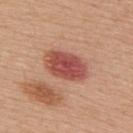This is a white-light tile. A close-up tile cropped from a whole-body skin photograph, about 15 mm across. Measured at roughly 5.5 mm in maximum diameter. Located on the back. The patient is a male aged 53–57. The total-body-photography lesion software estimated an outline eccentricity of about 0.8 (0 = round, 1 = elongated) and a shape-asymmetry score of about 0.15 (0 = symmetric).